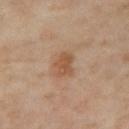Part of a total-body skin-imaging series; this lesion was reviewed on a skin check and was not flagged for biopsy. Captured under cross-polarized illumination. An algorithmic analysis of the crop reported a border-irregularity index near 3.5/10, internal color variation of about 2 on a 0–10 scale, and a peripheral color-asymmetry measure near 0.5. The subject is a female approximately 50 years of age. The recorded lesion diameter is about 3 mm. A close-up tile cropped from a whole-body skin photograph, about 15 mm across. The lesion is located on the right thigh.